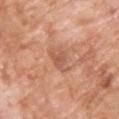– biopsy status: imaged on a skin check; not biopsied
– site: the front of the torso
– patient: male, in their 60s
– imaging modality: 15 mm crop, total-body photography